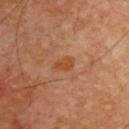• biopsy status — total-body-photography surveillance lesion; no biopsy
• body site — the upper back
• lesion diameter — about 2.5 mm
• acquisition — ~15 mm crop, total-body skin-cancer survey
• image-analysis metrics — a lesion area of about 3.5 mm², a shape eccentricity near 0.8, and a shape-asymmetry score of about 0.25 (0 = symmetric); a mean CIELAB color near L≈47 a*≈24 b*≈37, about 7 CIELAB-L* units darker than the surrounding skin, and a normalized border contrast of about 6.5; a border-irregularity index near 2.5/10, a color-variation rating of about 2/10, and radial color variation of about 0.5; a classifier nevus-likeness of about 5/100 and a lesion-detection confidence of about 100/100
• patient — male, roughly 60 years of age
• illumination — cross-polarized